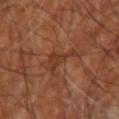This lesion was catalogued during total-body skin photography and was not selected for biopsy. From the right upper arm. Longest diameter approximately 3.5 mm. Captured under cross-polarized illumination. Cropped from a total-body skin-imaging series; the visible field is about 15 mm. A patient about 65 years old.